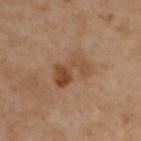Captured during whole-body skin photography for melanoma surveillance; the lesion was not biopsied. From the upper back. Longest diameter approximately 4.5 mm. A male patient, in their mid-50s. The tile uses cross-polarized illumination. A lesion tile, about 15 mm wide, cut from a 3D total-body photograph. The total-body-photography lesion software estimated a footprint of about 10 mm² and a symmetry-axis asymmetry near 0.3.